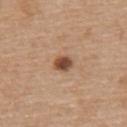No biopsy was performed on this lesion — it was imaged during a full skin examination and was not determined to be concerning. This image is a 15 mm lesion crop taken from a total-body photograph. The lesion is on the mid back. A male subject, in their 70s. Imaged with white-light lighting.